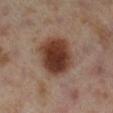lesion size — ~6 mm (longest diameter) | tile lighting — cross-polarized illumination | automated metrics — an average lesion color of about L≈38 a*≈20 b*≈26 (CIELAB), roughly 15 lightness units darker than nearby skin, and a lesion-to-skin contrast of about 12.5 (normalized; higher = more distinct); a nevus-likeness score of about 100/100 | image source — total-body-photography crop, ~15 mm field of view | site — the right lower leg | subject — female, approximately 40 years of age.